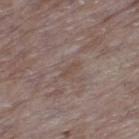Impression:
Imaged during a routine full-body skin examination; the lesion was not biopsied and no histopathology is available.
Clinical summary:
Approximately 2.5 mm at its widest. An algorithmic analysis of the crop reported a lesion color around L≈47 a*≈14 b*≈21 in CIELAB, roughly 5 lightness units darker than nearby skin, and a normalized border contrast of about 5. A region of skin cropped from a whole-body photographic capture, roughly 15 mm wide. From the leg. The patient is a female aged around 85 or older. This is a white-light tile.